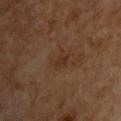Assessment: The lesion was tiled from a total-body skin photograph and was not biopsied. Background: A male patient roughly 65 years of age. Imaged with cross-polarized lighting. A 15 mm close-up extracted from a 3D total-body photography capture. The lesion's longest dimension is about 2.5 mm.